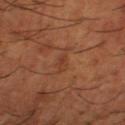The lesion was tiled from a total-body skin photograph and was not biopsied.
A close-up tile cropped from a whole-body skin photograph, about 15 mm across.
The lesion is located on the right thigh.
The patient is a male aged 63–67.